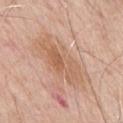notes: total-body-photography surveillance lesion; no biopsy | illumination: white-light illumination | imaging modality: 15 mm crop, total-body photography | patient: male, aged around 65 | anatomic site: the chest | automated metrics: a lesion area of about 16 mm², an eccentricity of roughly 0.9, and a shape-asymmetry score of about 0.25 (0 = symmetric); a mean CIELAB color near L≈61 a*≈20 b*≈32 and a normalized border contrast of about 6.5; a peripheral color-asymmetry measure near 1.5 | lesion size: ~7 mm (longest diameter).